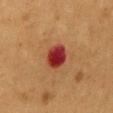imaging modality: ~15 mm tile from a whole-body skin photo
image-analysis metrics: a lesion area of about 7.5 mm² and an outline eccentricity of about 0.5 (0 = round, 1 = elongated); a border-irregularity index near 1/10 and peripheral color asymmetry of about 1
site: the mid back
size: ≈3 mm
subject: male, approximately 55 years of age
illumination: cross-polarized illumination